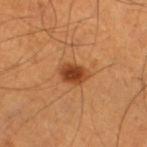Impression:
The lesion was tiled from a total-body skin photograph and was not biopsied.